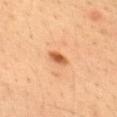size = ~2.5 mm (longest diameter) | body site = the upper back | imaging modality = ~15 mm tile from a whole-body skin photo | illumination = cross-polarized illumination | automated lesion analysis = a lesion area of about 3 mm² and two-axis asymmetry of about 0.2 | subject = male, aged 33 to 37.